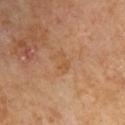No biopsy was performed on this lesion — it was imaged during a full skin examination and was not determined to be concerning. The total-body-photography lesion software estimated a lesion area of about 3.5 mm², a shape eccentricity near 0.85, and a shape-asymmetry score of about 0.25 (0 = symmetric). The analysis additionally found a within-lesion color-variation index near 1.5/10. The software also gave a nevus-likeness score of about 0/100 and a detector confidence of about 100 out of 100 that the crop contains a lesion. About 2.5 mm across. Captured under cross-polarized illumination. A male patient aged 68 to 72. On the right upper arm. Cropped from a whole-body photographic skin survey; the tile spans about 15 mm.